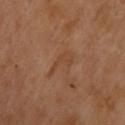biopsy status — imaged on a skin check; not biopsied
image source — total-body-photography crop, ~15 mm field of view
patient — male, aged around 70
anatomic site — the upper back